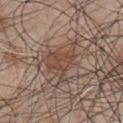The lesion was tiled from a total-body skin photograph and was not biopsied. A male patient, about 50 years old. Cropped from a whole-body photographic skin survey; the tile spans about 15 mm. Located on the front of the torso.